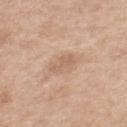biopsy status: catalogued during a skin exam; not biopsied
anatomic site: the mid back
image source: total-body-photography crop, ~15 mm field of view
automated lesion analysis: a footprint of about 3.5 mm², an outline eccentricity of about 0.8 (0 = round, 1 = elongated), and a symmetry-axis asymmetry near 0.3; about 8 CIELAB-L* units darker than the surrounding skin and a normalized lesion–skin contrast near 5; a border-irregularity rating of about 4/10, a color-variation rating of about 1/10, and radial color variation of about 0.5
illumination: white-light illumination
patient: female, aged around 40
lesion size: ≈3 mm